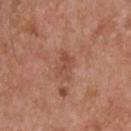Recorded during total-body skin imaging; not selected for excision or biopsy. A male subject, in their mid- to late 50s. A 15 mm close-up extracted from a 3D total-body photography capture. The tile uses white-light illumination. The lesion-visualizer software estimated an area of roughly 5 mm², a shape eccentricity near 0.8, and a symmetry-axis asymmetry near 0.35. It also reported border irregularity of about 4.5 on a 0–10 scale, a within-lesion color-variation index near 2.5/10, and radial color variation of about 1. The lesion is on the upper back. Longest diameter approximately 3 mm.